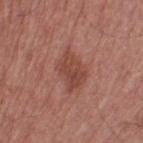| feature | finding |
|---|---|
| image | 15 mm crop, total-body photography |
| anatomic site | the leg |
| size | about 4.5 mm |
| patient | male, roughly 70 years of age |